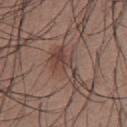| feature | finding |
|---|---|
| workup | total-body-photography surveillance lesion; no biopsy |
| tile lighting | white-light illumination |
| lesion size | about 5 mm |
| location | the chest |
| imaging modality | total-body-photography crop, ~15 mm field of view |
| patient | male, aged around 35 |